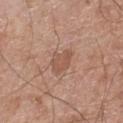notes = no biopsy performed (imaged during a skin exam)
subject = male, aged 58 to 62
anatomic site = the right lower leg
illumination = white-light
image source = ~15 mm crop, total-body skin-cancer survey